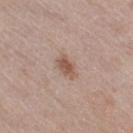follow-up: catalogued during a skin exam; not biopsied | image source: 15 mm crop, total-body photography | patient: female, aged 38–42 | body site: the right thigh.